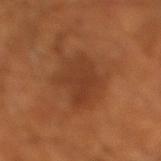Notes:
* biopsy status: no biopsy performed (imaged during a skin exam)